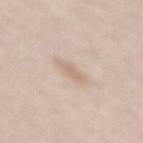Notes:
– biopsy status: imaged on a skin check; not biopsied
– patient: female, approximately 60 years of age
– imaging modality: ~15 mm crop, total-body skin-cancer survey
– lesion diameter: ~3.5 mm (longest diameter)
– lighting: white-light illumination
– anatomic site: the mid back